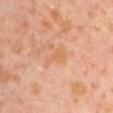Assessment: The lesion was tiled from a total-body skin photograph and was not biopsied. Background: A male subject, about 30 years old. From the arm. A roughly 15 mm field-of-view crop from a total-body skin photograph.